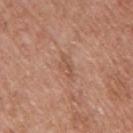Impression: This lesion was catalogued during total-body skin photography and was not selected for biopsy. Context: The lesion is located on the right upper arm. Captured under white-light illumination. Automated tile analysis of the lesion measured an eccentricity of roughly 0.85 and two-axis asymmetry of about 0.25. It also reported a lesion color around L≈54 a*≈22 b*≈30 in CIELAB, about 7 CIELAB-L* units darker than the surrounding skin, and a normalized border contrast of about 5. It also reported a border-irregularity index near 3/10, a within-lesion color-variation index near 2/10, and a peripheral color-asymmetry measure near 0.5. And it measured a classifier nevus-likeness of about 0/100 and lesion-presence confidence of about 100/100. The patient is a female aged 58–62. Measured at roughly 3 mm in maximum diameter. A 15 mm crop from a total-body photograph taken for skin-cancer surveillance.